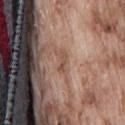Clinical impression:
This lesion was catalogued during total-body skin photography and was not selected for biopsy.
Image and clinical context:
About 2.5 mm across. Cropped from a whole-body photographic skin survey; the tile spans about 15 mm. Captured under white-light illumination. The lesion is on the lower back. Automated tile analysis of the lesion measured about 8 CIELAB-L* units darker than the surrounding skin and a normalized border contrast of about 6. And it measured a classifier nevus-likeness of about 0/100 and lesion-presence confidence of about 80/100. A male patient, in their mid- to late 70s.